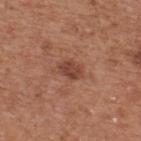| field | value |
|---|---|
| patient | male, approximately 65 years of age |
| lighting | white-light |
| site | the back |
| imaging modality | 15 mm crop, total-body photography |